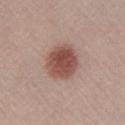Imaged during a routine full-body skin examination; the lesion was not biopsied and no histopathology is available. Measured at roughly 4.5 mm in maximum diameter. From the right lower leg. A close-up tile cropped from a whole-body skin photograph, about 15 mm across. Captured under white-light illumination. A female subject, aged 18 to 22.